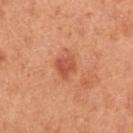workup = total-body-photography surveillance lesion; no biopsy | acquisition = 15 mm crop, total-body photography | patient = female, aged 38–42 | site = the left upper arm.